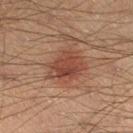Recorded during total-body skin imaging; not selected for excision or biopsy.
The subject is a male aged approximately 40.
This is a cross-polarized tile.
The lesion's longest dimension is about 4.5 mm.
The lesion is on the left lower leg.
A roughly 15 mm field-of-view crop from a total-body skin photograph.